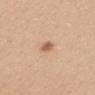<lesion>
  <biopsy_status>not biopsied; imaged during a skin examination</biopsy_status>
  <site>upper back</site>
  <patient>
    <sex>female</sex>
    <age_approx>55</age_approx>
  </patient>
  <image>
    <source>total-body photography crop</source>
    <field_of_view_mm>15</field_of_view_mm>
  </image>
</lesion>